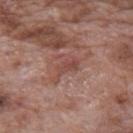{"image": {"source": "total-body photography crop", "field_of_view_mm": 15}, "automated_metrics": {"area_mm2_approx": 6.0, "eccentricity": 0.75, "shape_asymmetry": 0.65, "cielab_L": 47, "cielab_a": 23, "cielab_b": 24, "border_irregularity_0_10": 8.0, "peripheral_color_asymmetry": 1.0, "nevus_likeness_0_100": 0}, "site": "mid back", "patient": {"sex": "male", "age_approx": 70}, "lesion_size": {"long_diameter_mm_approx": 4.0}}